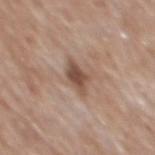workup: no biopsy performed (imaged during a skin exam)
body site: the mid back
TBP lesion metrics: a mean CIELAB color near L≈49 a*≈18 b*≈26, roughly 12 lightness units darker than nearby skin, and a normalized lesion–skin contrast near 9; border irregularity of about 3.5 on a 0–10 scale, internal color variation of about 2 on a 0–10 scale, and a peripheral color-asymmetry measure near 0.5; a classifier nevus-likeness of about 30/100 and lesion-presence confidence of about 100/100
lesion diameter: ~3.5 mm (longest diameter)
image source: total-body-photography crop, ~15 mm field of view
patient: male, roughly 60 years of age